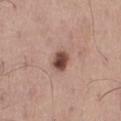follow-up = total-body-photography surveillance lesion; no biopsy | diameter = about 2.5 mm | body site = the right thigh | image = ~15 mm crop, total-body skin-cancer survey | tile lighting = white-light | subject = male, aged around 55.